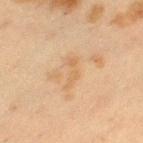<tbp_lesion>
  <biopsy_status>not biopsied; imaged during a skin examination</biopsy_status>
</tbp_lesion>